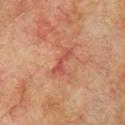biopsy status=catalogued during a skin exam; not biopsied | illumination=cross-polarized | acquisition=~15 mm tile from a whole-body skin photo | subject=male, aged 73–77 | body site=the left upper arm | TBP lesion metrics=an area of roughly 3 mm², an eccentricity of roughly 0.95, and two-axis asymmetry of about 0.65; about 7 CIELAB-L* units darker than the surrounding skin and a normalized lesion–skin contrast near 6 | lesion size=about 3.5 mm.